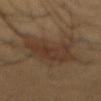Case summary:
* notes — total-body-photography surveillance lesion; no biopsy
* tile lighting — cross-polarized illumination
* diameter — about 8 mm
* body site — the front of the torso
* acquisition — ~15 mm tile from a whole-body skin photo
* subject — male, roughly 45 years of age